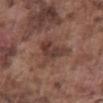Imaged during a routine full-body skin examination; the lesion was not biopsied and no histopathology is available.
This is a white-light tile.
The total-body-photography lesion software estimated a lesion color around L≈40 a*≈19 b*≈23 in CIELAB and a normalized lesion–skin contrast near 7. The software also gave a nevus-likeness score of about 0/100 and a detector confidence of about 100 out of 100 that the crop contains a lesion.
On the abdomen.
The subject is a male approximately 75 years of age.
A 15 mm close-up tile from a total-body photography series done for melanoma screening.
Longest diameter approximately 5 mm.